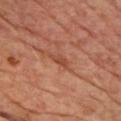{"biopsy_status": "not biopsied; imaged during a skin examination", "site": "chest", "automated_metrics": {"cielab_L": 45, "cielab_a": 26, "cielab_b": 30, "vs_skin_darker_L": 8.0, "vs_skin_contrast_norm": 6.0, "nevus_likeness_0_100": 0}, "image": {"source": "total-body photography crop", "field_of_view_mm": 15}, "lesion_size": {"long_diameter_mm_approx": 3.0}, "patient": {"sex": "male", "age_approx": 65}, "lighting": "cross-polarized"}